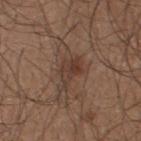Assessment: The lesion was tiled from a total-body skin photograph and was not biopsied. Clinical summary: Located on the back. Measured at roughly 3.5 mm in maximum diameter. Automated tile analysis of the lesion measured a mean CIELAB color near L≈37 a*≈17 b*≈24, roughly 7 lightness units darker than nearby skin, and a lesion-to-skin contrast of about 6.5 (normalized; higher = more distinct). A 15 mm close-up extracted from a 3D total-body photography capture. The subject is a male approximately 25 years of age.